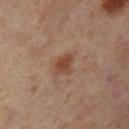The lesion is on the left lower leg. The patient is a female aged approximately 55. A roughly 15 mm field-of-view crop from a total-body skin photograph.